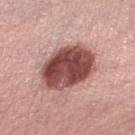Captured during whole-body skin photography for melanoma surveillance; the lesion was not biopsied. Captured under white-light illumination. From the left lower leg. The patient is a female aged 63 to 67. The total-body-photography lesion software estimated a lesion area of about 24 mm², an outline eccentricity of about 0.7 (0 = round, 1 = elongated), and two-axis asymmetry of about 0.1. It also reported a border-irregularity rating of about 1.5/10, a within-lesion color-variation index near 6.5/10, and radial color variation of about 2.5. The software also gave a classifier nevus-likeness of about 70/100. A region of skin cropped from a whole-body photographic capture, roughly 15 mm wide.